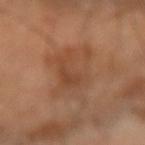Assessment: The lesion was photographed on a routine skin check and not biopsied; there is no pathology result. Clinical summary: This image is a 15 mm lesion crop taken from a total-body photograph. From the right forearm. The subject is a male aged 63 to 67.